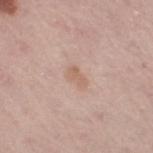{
  "biopsy_status": "not biopsied; imaged during a skin examination",
  "lighting": "white-light",
  "image": {
    "source": "total-body photography crop",
    "field_of_view_mm": 15
  },
  "site": "right thigh",
  "patient": {
    "sex": "female",
    "age_approx": 65
  },
  "automated_metrics": {
    "area_mm2_approx": 3.5,
    "eccentricity": 0.85,
    "cielab_L": 62,
    "cielab_a": 18,
    "cielab_b": 28,
    "vs_skin_darker_L": 7.0,
    "vs_skin_contrast_norm": 5.5,
    "border_irregularity_0_10": 3.0,
    "color_variation_0_10": 1.0,
    "peripheral_color_asymmetry": 0.5,
    "nevus_likeness_0_100": 0
  }
}